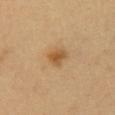<case>
  <biopsy_status>not biopsied; imaged during a skin examination</biopsy_status>
  <patient>
    <sex>female</sex>
    <age_approx>35</age_approx>
  </patient>
  <site>front of the torso</site>
  <image>
    <source>total-body photography crop</source>
    <field_of_view_mm>15</field_of_view_mm>
  </image>
</case>